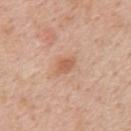biopsy status = total-body-photography surveillance lesion; no biopsy
site = the upper back
patient = male, aged 48–52
diameter = ≈3 mm
imaging modality = ~15 mm tile from a whole-body skin photo
lighting = white-light illumination